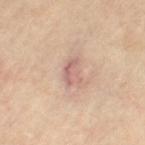Part of a total-body skin-imaging series; this lesion was reviewed on a skin check and was not flagged for biopsy. Longest diameter approximately 3 mm. On the left thigh. This is a cross-polarized tile. Automated image analysis of the tile measured a classifier nevus-likeness of about 0/100. A female patient, about 65 years old. A 15 mm close-up extracted from a 3D total-body photography capture.